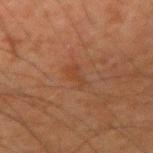{
  "biopsy_status": "not biopsied; imaged during a skin examination",
  "image": {
    "source": "total-body photography crop",
    "field_of_view_mm": 15
  },
  "site": "right upper arm",
  "automated_metrics": {
    "nevus_likeness_0_100": 0,
    "lesion_detection_confidence_0_100": 100
  },
  "lesion_size": {
    "long_diameter_mm_approx": 3.0
  },
  "lighting": "cross-polarized",
  "patient": {
    "sex": "male",
    "age_approx": 35
  }
}